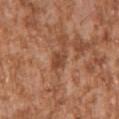biopsy status: no biopsy performed (imaged during a skin exam)
image: total-body-photography crop, ~15 mm field of view
subject: male, approximately 45 years of age
anatomic site: the left upper arm